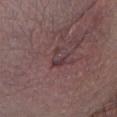biopsy status = no biopsy performed (imaged during a skin exam) | patient = male, aged approximately 55 | body site = the abdomen | image = 15 mm crop, total-body photography.